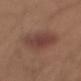Assessment: Imaged during a routine full-body skin examination; the lesion was not biopsied and no histopathology is available. Clinical summary: Captured under white-light illumination. A male subject, aged approximately 30. A 15 mm crop from a total-body photograph taken for skin-cancer surveillance. Located on the arm. Approximately 5.5 mm at its widest.